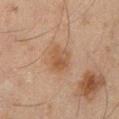Q: Is there a histopathology result?
A: catalogued during a skin exam; not biopsied
Q: Patient demographics?
A: male, aged around 45
Q: What is the imaging modality?
A: ~15 mm crop, total-body skin-cancer survey
Q: How was the tile lit?
A: cross-polarized
Q: Lesion size?
A: about 3.5 mm
Q: What is the anatomic site?
A: the left lower leg